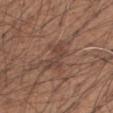Recorded during total-body skin imaging; not selected for excision or biopsy. From the left forearm. Captured under white-light illumination. A male patient, in their mid- to late 50s. Approximately 4.5 mm at its widest. Cropped from a whole-body photographic skin survey; the tile spans about 15 mm. An algorithmic analysis of the crop reported a lesion area of about 8 mm², an eccentricity of roughly 0.85, and a symmetry-axis asymmetry near 0.35. And it measured an average lesion color of about L≈43 a*≈18 b*≈25 (CIELAB), about 7 CIELAB-L* units darker than the surrounding skin, and a normalized border contrast of about 5.5. It also reported radial color variation of about 1.5. The analysis additionally found an automated nevus-likeness rating near 0 out of 100 and lesion-presence confidence of about 65/100.